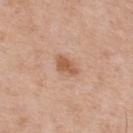The lesion was tiled from a total-body skin photograph and was not biopsied.
A male subject aged around 55.
Cropped from a total-body skin-imaging series; the visible field is about 15 mm.
Longest diameter approximately 3 mm.
Located on the upper back.